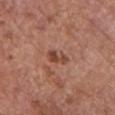Captured during whole-body skin photography for melanoma surveillance; the lesion was not biopsied.
On the chest.
The lesion-visualizer software estimated an area of roughly 3.5 mm² and a shape eccentricity near 0.9. The analysis additionally found a mean CIELAB color near L≈45 a*≈24 b*≈29, roughly 10 lightness units darker than nearby skin, and a lesion-to-skin contrast of about 8 (normalized; higher = more distinct). And it measured lesion-presence confidence of about 100/100.
The tile uses white-light illumination.
A female subject, aged 63 to 67.
A close-up tile cropped from a whole-body skin photograph, about 15 mm across.